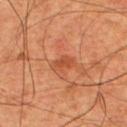biopsy_status: not biopsied; imaged during a skin examination
patient:
  sex: male
  age_approx: 55
lighting: cross-polarized
lesion_size:
  long_diameter_mm_approx: 3.0
image:
  source: total-body photography crop
  field_of_view_mm: 15
site: chest